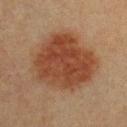| key | value |
|---|---|
| workup | total-body-photography surveillance lesion; no biopsy |